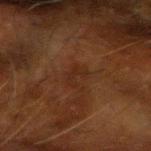subject = male, aged around 65; lighting = cross-polarized illumination; acquisition = total-body-photography crop, ~15 mm field of view; size = ~2.5 mm (longest diameter); anatomic site = the left forearm.